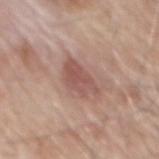Q: Is there a histopathology result?
A: total-body-photography surveillance lesion; no biopsy